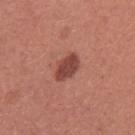Case summary:
* follow-up — no biopsy performed (imaged during a skin exam)
* acquisition — ~15 mm tile from a whole-body skin photo
* site — the left upper arm
* subject — female, in their mid-20s